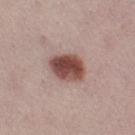biopsy status: total-body-photography surveillance lesion; no biopsy | image: ~15 mm crop, total-body skin-cancer survey | body site: the right thigh | automated lesion analysis: a lesion color around L≈47 a*≈22 b*≈23 in CIELAB, roughly 17 lightness units darker than nearby skin, and a normalized border contrast of about 12; a border-irregularity index near 1.5/10 and a within-lesion color-variation index near 6.5/10; a nevus-likeness score of about 100/100 and lesion-presence confidence of about 100/100 | patient: female, aged approximately 25 | lesion diameter: about 4 mm.